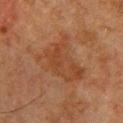Imaged during a routine full-body skin examination; the lesion was not biopsied and no histopathology is available.
Located on the chest.
Measured at roughly 6.5 mm in maximum diameter.
This is a cross-polarized tile.
A male patient roughly 80 years of age.
A roughly 15 mm field-of-view crop from a total-body skin photograph.